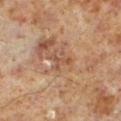Recorded during total-body skin imaging; not selected for excision or biopsy. The lesion-visualizer software estimated a lesion color around L≈48 a*≈20 b*≈30 in CIELAB, roughly 8 lightness units darker than nearby skin, and a normalized border contrast of about 6. The software also gave a border-irregularity index near 8/10 and a within-lesion color-variation index near 0/10. The software also gave a classifier nevus-likeness of about 0/100 and a lesion-detection confidence of about 85/100. The lesion is located on the leg. The subject is a male aged 58 to 62. The tile uses cross-polarized illumination. A 15 mm crop from a total-body photograph taken for skin-cancer surveillance. Longest diameter approximately 3 mm.